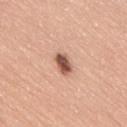Acquisition and patient details:
The lesion is on the back. A roughly 15 mm field-of-view crop from a total-body skin photograph. Captured under white-light illumination. About 3 mm across. A male subject, approximately 60 years of age.